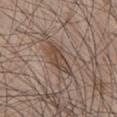Located on the chest. This is a white-light tile. A male subject, aged 48–52. Cropped from a total-body skin-imaging series; the visible field is about 15 mm. The recorded lesion diameter is about 5 mm.